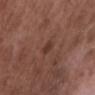Q: Was a biopsy performed?
A: total-body-photography surveillance lesion; no biopsy
Q: What are the patient's age and sex?
A: male, aged around 50
Q: Where on the body is the lesion?
A: the chest
Q: What is the imaging modality?
A: ~15 mm crop, total-body skin-cancer survey